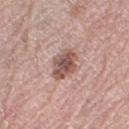Findings:
– follow-up: catalogued during a skin exam; not biopsied
– acquisition: total-body-photography crop, ~15 mm field of view
– site: the left thigh
– patient: female, in their mid-60s